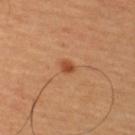Clinical impression:
Imaged during a routine full-body skin examination; the lesion was not biopsied and no histopathology is available.
Image and clinical context:
This image is a 15 mm lesion crop taken from a total-body photograph. The tile uses cross-polarized illumination. A male subject, aged around 65. Located on the upper back. The recorded lesion diameter is about 2.5 mm.